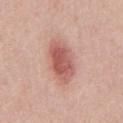Captured during whole-body skin photography for melanoma surveillance; the lesion was not biopsied. A 15 mm close-up tile from a total-body photography series done for melanoma screening. A male patient about 55 years old. The lesion is located on the chest.